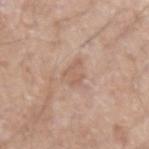biopsy status — catalogued during a skin exam; not biopsied | body site — the left forearm | image-analysis metrics — a footprint of about 4.5 mm², a shape eccentricity near 0.7, and a shape-asymmetry score of about 0.4 (0 = symmetric); roughly 7 lightness units darker than nearby skin and a normalized border contrast of about 5; border irregularity of about 4.5 on a 0–10 scale and radial color variation of about 0.5; a nevus-likeness score of about 0/100 and lesion-presence confidence of about 100/100 | lesion size — ~3 mm (longest diameter) | subject — male, in their 70s | acquisition — ~15 mm crop, total-body skin-cancer survey.